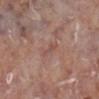Captured during whole-body skin photography for melanoma surveillance; the lesion was not biopsied.
The lesion is located on the left lower leg.
A 15 mm close-up extracted from a 3D total-body photography capture.
The subject is a female aged around 75.